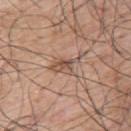Q: Was a biopsy performed?
A: imaged on a skin check; not biopsied
Q: What lighting was used for the tile?
A: white-light
Q: Lesion size?
A: about 4 mm
Q: What did automated image analysis measure?
A: roughly 10 lightness units darker than nearby skin and a lesion-to-skin contrast of about 7 (normalized; higher = more distinct)
Q: What are the patient's age and sex?
A: male, aged approximately 70
Q: What kind of image is this?
A: total-body-photography crop, ~15 mm field of view
Q: Lesion location?
A: the upper back